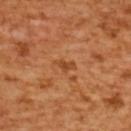Findings:
- workup · catalogued during a skin exam; not biopsied
- lighting · cross-polarized
- diameter · ≈2.5 mm
- subject · female, about 55 years old
- anatomic site · the upper back
- acquisition · ~15 mm tile from a whole-body skin photo